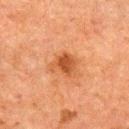Q: Was a biopsy performed?
A: no biopsy performed (imaged during a skin exam)
Q: What did automated image analysis measure?
A: a lesion color around L≈42 a*≈25 b*≈34 in CIELAB, a lesion–skin lightness drop of about 9, and a normalized border contrast of about 7.5
Q: Lesion size?
A: about 3 mm
Q: Lesion location?
A: the right upper arm
Q: Illumination type?
A: cross-polarized illumination
Q: What are the patient's age and sex?
A: male, in their 60s
Q: How was this image acquired?
A: ~15 mm crop, total-body skin-cancer survey